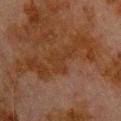biopsy status — total-body-photography surveillance lesion; no biopsy | lesion size — ≈4 mm | subject — male, aged around 80 | image-analysis metrics — an area of roughly 5.5 mm², an outline eccentricity of about 0.85 (0 = round, 1 = elongated), and a symmetry-axis asymmetry near 0.7; internal color variation of about 1 on a 0–10 scale and peripheral color asymmetry of about 0.5; a classifier nevus-likeness of about 0/100 and a lesion-detection confidence of about 95/100 | location — the upper back | image — ~15 mm tile from a whole-body skin photo | illumination — cross-polarized illumination.